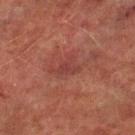<lesion>
  <biopsy_status>not biopsied; imaged during a skin examination</biopsy_status>
  <site>left lower leg</site>
  <automated_metrics>
    <area_mm2_approx>4.5</area_mm2_approx>
    <eccentricity>0.85</eccentricity>
    <cielab_L>33</cielab_L>
    <cielab_a>24</cielab_a>
    <cielab_b>22</cielab_b>
  </automated_metrics>
  <lighting>cross-polarized</lighting>
  <image>
    <source>total-body photography crop</source>
    <field_of_view_mm>15</field_of_view_mm>
  </image>
  <patient>
    <sex>male</sex>
    <age_approx>75</age_approx>
  </patient>
</lesion>